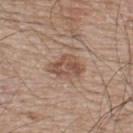Findings:
- follow-up — no biopsy performed (imaged during a skin exam)
- lesion diameter — ≈4 mm
- image source — ~15 mm tile from a whole-body skin photo
- lighting — white-light illumination
- anatomic site — the upper back
- subject — male, aged approximately 65